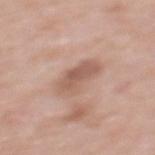Part of a total-body skin-imaging series; this lesion was reviewed on a skin check and was not flagged for biopsy.
A lesion tile, about 15 mm wide, cut from a 3D total-body photograph.
A female subject aged approximately 40.
The lesion is located on the upper back.